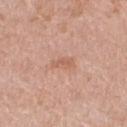| key | value |
|---|---|
| biopsy status | total-body-photography surveillance lesion; no biopsy |
| acquisition | ~15 mm tile from a whole-body skin photo |
| tile lighting | white-light |
| size | ≈2.5 mm |
| patient | female, approximately 70 years of age |
| anatomic site | the right thigh |
| image-analysis metrics | an area of roughly 3 mm², an outline eccentricity of about 0.9 (0 = round, 1 = elongated), and a symmetry-axis asymmetry near 0.45 |